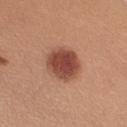No biopsy was performed on this lesion — it was imaged during a full skin examination and was not determined to be concerning. Cropped from a total-body skin-imaging series; the visible field is about 15 mm. Located on the right upper arm. A female subject, aged approximately 45.